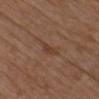Part of a total-body skin-imaging series; this lesion was reviewed on a skin check and was not flagged for biopsy.
The lesion is on the chest.
A male subject in their mid-70s.
Approximately 2.5 mm at its widest.
Imaged with white-light lighting.
A region of skin cropped from a whole-body photographic capture, roughly 15 mm wide.